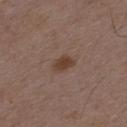follow-up: total-body-photography surveillance lesion; no biopsy | lighting: white-light illumination | image source: ~15 mm crop, total-body skin-cancer survey | patient: male, aged 48–52 | anatomic site: the mid back | TBP lesion metrics: a footprint of about 4.5 mm², an outline eccentricity of about 0.7 (0 = round, 1 = elongated), and two-axis asymmetry of about 0.25; a lesion color around L≈40 a*≈17 b*≈25 in CIELAB, roughly 8 lightness units darker than nearby skin, and a lesion-to-skin contrast of about 8 (normalized; higher = more distinct); border irregularity of about 2 on a 0–10 scale, a color-variation rating of about 1.5/10, and peripheral color asymmetry of about 0.5 | lesion diameter: ~2.5 mm (longest diameter).